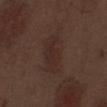This lesion was catalogued during total-body skin photography and was not selected for biopsy.
The tile uses white-light illumination.
A male patient, aged 68 to 72.
This image is a 15 mm lesion crop taken from a total-body photograph.
Located on the abdomen.